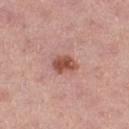Assessment:
Imaged during a routine full-body skin examination; the lesion was not biopsied and no histopathology is available.
Clinical summary:
From the right thigh. Captured under white-light illumination. Longest diameter approximately 3 mm. A female patient, aged around 45. Cropped from a total-body skin-imaging series; the visible field is about 15 mm.